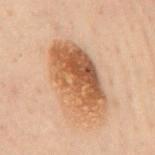This lesion was catalogued during total-body skin photography and was not selected for biopsy. The tile uses cross-polarized illumination. The recorded lesion diameter is about 8.5 mm. A male subject aged 48 to 52. From the mid back. A 15 mm close-up extracted from a 3D total-body photography capture.